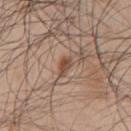Impression: This lesion was catalogued during total-body skin photography and was not selected for biopsy. Image and clinical context: The recorded lesion diameter is about 2.5 mm. Located on the chest. A male patient aged approximately 45. Imaged with white-light lighting. A region of skin cropped from a whole-body photographic capture, roughly 15 mm wide.